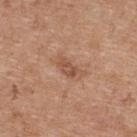notes — imaged on a skin check; not biopsied | image — total-body-photography crop, ~15 mm field of view | subject — female, aged 38–42 | image-analysis metrics — a footprint of about 4.5 mm², an outline eccentricity of about 0.85 (0 = round, 1 = elongated), and a shape-asymmetry score of about 0.35 (0 = symmetric); a color-variation rating of about 2.5/10 and a peripheral color-asymmetry measure near 0.5 | lighting — white-light | location — the upper back | lesion size — ~3.5 mm (longest diameter).